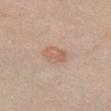The lesion was photographed on a routine skin check and not biopsied; there is no pathology result. A close-up tile cropped from a whole-body skin photograph, about 15 mm across. The lesion is located on the front of the torso. Captured under white-light illumination. A female subject about 35 years old. Approximately 3.5 mm at its widest. Automated tile analysis of the lesion measured a shape eccentricity near 0.7 and two-axis asymmetry of about 0.2. And it measured a lesion color around L≈61 a*≈19 b*≈30 in CIELAB and a normalized lesion–skin contrast near 5.5. It also reported a border-irregularity rating of about 2/10 and a peripheral color-asymmetry measure near 1.